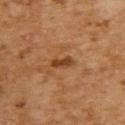This lesion was catalogued during total-body skin photography and was not selected for biopsy. A female patient about 60 years old. Automated image analysis of the tile measured a lesion color around L≈37 a*≈21 b*≈33 in CIELAB, a lesion–skin lightness drop of about 9, and a lesion-to-skin contrast of about 8.5 (normalized; higher = more distinct). It also reported border irregularity of about 3 on a 0–10 scale and internal color variation of about 0.5 on a 0–10 scale. The software also gave a nevus-likeness score of about 50/100 and lesion-presence confidence of about 100/100. The recorded lesion diameter is about 3 mm. From the upper back. Cropped from a total-body skin-imaging series; the visible field is about 15 mm.